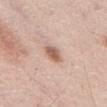Recorded during total-body skin imaging; not selected for excision or biopsy. The lesion is on the abdomen. A 15 mm close-up extracted from a 3D total-body photography capture. A male subject, aged approximately 55. This is a white-light tile. The total-body-photography lesion software estimated an area of roughly 5 mm² and a symmetry-axis asymmetry near 0.2. Approximately 3 mm at its widest.